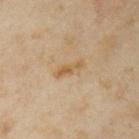Q: Was a biopsy performed?
A: catalogued during a skin exam; not biopsied
Q: Patient demographics?
A: female, aged approximately 35
Q: How was this image acquired?
A: ~15 mm tile from a whole-body skin photo
Q: How was the tile lit?
A: cross-polarized
Q: Lesion size?
A: ≈3.5 mm
Q: What is the anatomic site?
A: the left upper arm